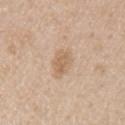Q: Who is the patient?
A: male, aged approximately 55
Q: What did automated image analysis measure?
A: a lesion color around L≈64 a*≈16 b*≈32 in CIELAB and a lesion–skin lightness drop of about 8; a border-irregularity index near 2/10, a within-lesion color-variation index near 2.5/10, and radial color variation of about 1
Q: How was this image acquired?
A: ~15 mm tile from a whole-body skin photo
Q: What is the lesion's diameter?
A: ~4 mm (longest diameter)
Q: Illumination type?
A: white-light
Q: Lesion location?
A: the right upper arm